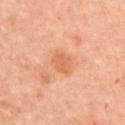  biopsy_status: not biopsied; imaged during a skin examination
  patient:
    sex: female
    age_approx: 50
  lesion_size:
    long_diameter_mm_approx: 2.5
  image:
    source: total-body photography crop
    field_of_view_mm: 15
  site: left upper arm